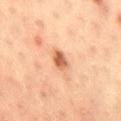– biopsy status · imaged on a skin check; not biopsied
– diameter · about 3 mm
– location · the right thigh
– acquisition · total-body-photography crop, ~15 mm field of view
– illumination · cross-polarized
– patient · male, in their mid-30s